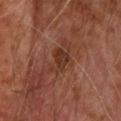Impression:
The lesion was tiled from a total-body skin photograph and was not biopsied.